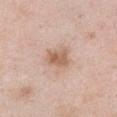Part of a total-body skin-imaging series; this lesion was reviewed on a skin check and was not flagged for biopsy.
Measured at roughly 3 mm in maximum diameter.
From the chest.
A female subject, roughly 50 years of age.
A roughly 15 mm field-of-view crop from a total-body skin photograph.
The total-body-photography lesion software estimated an area of roughly 5.5 mm² and an outline eccentricity of about 0.6 (0 = round, 1 = elongated). The analysis additionally found a mean CIELAB color near L≈60 a*≈19 b*≈30, about 11 CIELAB-L* units darker than the surrounding skin, and a normalized border contrast of about 7.5. It also reported a lesion-detection confidence of about 100/100.
The tile uses white-light illumination.